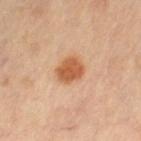• notes · catalogued during a skin exam; not biopsied
• illumination · cross-polarized
• imaging modality · ~15 mm crop, total-body skin-cancer survey
• patient · female, roughly 60 years of age
• body site · the right leg
• lesion diameter · ~3 mm (longest diameter)
• image-analysis metrics · a lesion area of about 7 mm² and a symmetry-axis asymmetry near 0.15; a classifier nevus-likeness of about 100/100 and a detector confidence of about 100 out of 100 that the crop contains a lesion